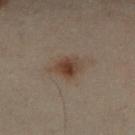Case summary:
- follow-up: imaged on a skin check; not biopsied
- size: about 3.5 mm
- site: the leg
- patient: male, about 50 years old
- acquisition: 15 mm crop, total-body photography